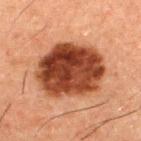Findings:
* size: ≈7.5 mm
* location: the upper back
* image: ~15 mm crop, total-body skin-cancer survey
* image-analysis metrics: an area of roughly 37 mm², a shape eccentricity near 0.6, and two-axis asymmetry of about 0.1
* subject: male, approximately 50 years of age
* lighting: cross-polarized